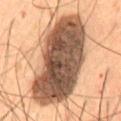Recorded during total-body skin imaging; not selected for excision or biopsy.
The recorded lesion diameter is about 12 mm.
A roughly 15 mm field-of-view crop from a total-body skin photograph.
The lesion-visualizer software estimated a footprint of about 60 mm² and a shape-asymmetry score of about 0.15 (0 = symmetric).
A male patient, aged around 55.
On the abdomen.